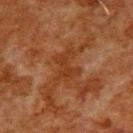Assessment: This lesion was catalogued during total-body skin photography and was not selected for biopsy. Background: A lesion tile, about 15 mm wide, cut from a 3D total-body photograph. From the upper back. The recorded lesion diameter is about 4 mm. A male patient, roughly 80 years of age.